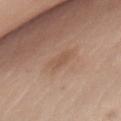Captured during whole-body skin photography for melanoma surveillance; the lesion was not biopsied.
A region of skin cropped from a whole-body photographic capture, roughly 15 mm wide.
A female patient, aged around 75.
Located on the chest.